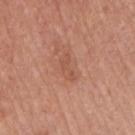– image source: ~15 mm crop, total-body skin-cancer survey
– location: the arm
– subject: male, roughly 65 years of age
– illumination: white-light